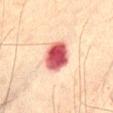Findings:
- biopsy status — imaged on a skin check; not biopsied
- subject — male, in their mid- to late 70s
- imaging modality — 15 mm crop, total-body photography
- anatomic site — the abdomen